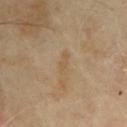Q: Where on the body is the lesion?
A: the left upper arm
Q: Automated lesion metrics?
A: a lesion area of about 2.5 mm² and a shape eccentricity near 0.95; a border-irregularity index near 4/10, a within-lesion color-variation index near 0/10, and radial color variation of about 0
Q: Who is the patient?
A: male, aged 63 to 67
Q: How was this image acquired?
A: ~15 mm crop, total-body skin-cancer survey
Q: What lighting was used for the tile?
A: cross-polarized
Q: Lesion size?
A: ~3 mm (longest diameter)